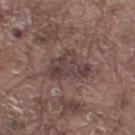The lesion was photographed on a routine skin check and not biopsied; there is no pathology result. Imaged with white-light lighting. This image is a 15 mm lesion crop taken from a total-body photograph. On the leg. A male patient, about 80 years old. The lesion-visualizer software estimated a lesion area of about 11 mm², a shape eccentricity near 0.8, and two-axis asymmetry of about 0.45. And it measured an average lesion color of about L≈40 a*≈16 b*≈18 (CIELAB), roughly 8 lightness units darker than nearby skin, and a normalized border contrast of about 7.5. The analysis additionally found a border-irregularity index near 5/10, a within-lesion color-variation index near 4.5/10, and a peripheral color-asymmetry measure near 1.5. It also reported a lesion-detection confidence of about 70/100.